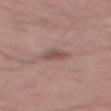The lesion was photographed on a routine skin check and not biopsied; there is no pathology result. A 15 mm close-up extracted from a 3D total-body photography capture. Automated tile analysis of the lesion measured a footprint of about 4 mm², an eccentricity of roughly 0.75, and a shape-asymmetry score of about 0.25 (0 = symmetric). And it measured a border-irregularity rating of about 2.5/10 and a peripheral color-asymmetry measure near 0.5. Approximately 3 mm at its widest. Located on the mid back. This is a white-light tile. The patient is a male approximately 55 years of age.